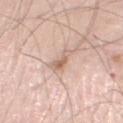Notes:
- workup · catalogued during a skin exam; not biopsied
- image · total-body-photography crop, ~15 mm field of view
- patient · male, aged 58 to 62
- site · the left lower leg
- tile lighting · white-light